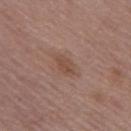{"biopsy_status": "not biopsied; imaged during a skin examination"}